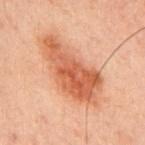This lesion was catalogued during total-body skin photography and was not selected for biopsy.
A 15 mm close-up extracted from a 3D total-body photography capture.
A male patient, approximately 60 years of age.
From the chest.
Imaged with cross-polarized lighting.